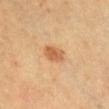Assessment: The lesion was photographed on a routine skin check and not biopsied; there is no pathology result. Clinical summary: From the chest. This is a cross-polarized tile. A male subject, aged approximately 40. A 15 mm close-up tile from a total-body photography series done for melanoma screening. The total-body-photography lesion software estimated a footprint of about 5 mm², an eccentricity of roughly 0.75, and two-axis asymmetry of about 0.15. And it measured border irregularity of about 1.5 on a 0–10 scale, a within-lesion color-variation index near 2.5/10, and peripheral color asymmetry of about 1. About 3 mm across.